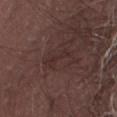Case summary:
* workup: no biopsy performed (imaged during a skin exam)
* illumination: white-light
* anatomic site: the leg
* subject: female, aged around 50
* image: ~15 mm crop, total-body skin-cancer survey
* diameter: ≈3.5 mm